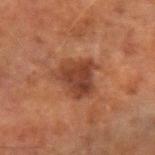Background:
Longest diameter approximately 5 mm. A male subject, approximately 60 years of age. A lesion tile, about 15 mm wide, cut from a 3D total-body photograph. The total-body-photography lesion software estimated a lesion area of about 13 mm², a shape eccentricity near 0.2, and a shape-asymmetry score of about 0.35 (0 = symmetric). It also reported a lesion color around L≈34 a*≈21 b*≈27 in CIELAB, about 9 CIELAB-L* units darker than the surrounding skin, and a normalized border contrast of about 8.5. It also reported a border-irregularity rating of about 4/10 and a color-variation rating of about 3.5/10. It also reported a detector confidence of about 100 out of 100 that the crop contains a lesion. The tile uses cross-polarized illumination. From the left forearm.